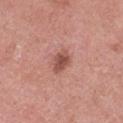The lesion was photographed on a routine skin check and not biopsied; there is no pathology result. About 2.5 mm across. From the left thigh. A female subject roughly 70 years of age. Imaged with white-light lighting. A 15 mm close-up extracted from a 3D total-body photography capture.